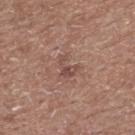Assessment: The lesion was tiled from a total-body skin photograph and was not biopsied. Background: A 15 mm crop from a total-body photograph taken for skin-cancer surveillance. The patient is a male approximately 60 years of age. Located on the upper back.